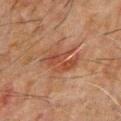follow-up: imaged on a skin check; not biopsied
illumination: cross-polarized illumination
image-analysis metrics: a lesion area of about 11 mm², an eccentricity of roughly 0.8, and a symmetry-axis asymmetry near 0.35; a border-irregularity index near 6.5/10, internal color variation of about 4 on a 0–10 scale, and radial color variation of about 1.5
site: the chest
patient: male, aged around 60
imaging modality: 15 mm crop, total-body photography